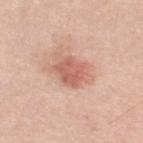{"biopsy_status": "not biopsied; imaged during a skin examination", "lighting": "white-light", "site": "left lower leg", "patient": {"sex": "male", "age_approx": 70}, "image": {"source": "total-body photography crop", "field_of_view_mm": 15}}